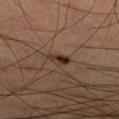Assessment: Recorded during total-body skin imaging; not selected for excision or biopsy. Context: Automated image analysis of the tile measured a lesion area of about 3.5 mm², an outline eccentricity of about 0.8 (0 = round, 1 = elongated), and a symmetry-axis asymmetry near 0.3. The software also gave a lesion color around L≈31 a*≈17 b*≈25 in CIELAB, roughly 11 lightness units darker than nearby skin, and a lesion-to-skin contrast of about 10 (normalized; higher = more distinct). The software also gave border irregularity of about 3 on a 0–10 scale and a within-lesion color-variation index near 5/10. And it measured a classifier nevus-likeness of about 100/100. The tile uses cross-polarized illumination. A roughly 15 mm field-of-view crop from a total-body skin photograph. Located on the right lower leg. A male patient, in their 60s. Measured at roughly 3 mm in maximum diameter.